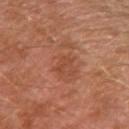notes: imaged on a skin check; not biopsied
subject: male, aged 28–32
location: the left upper arm
TBP lesion metrics: an area of roughly 2 mm² and an eccentricity of roughly 0.95; a border-irregularity rating of about 5.5/10, a within-lesion color-variation index near 0/10, and radial color variation of about 0
imaging modality: total-body-photography crop, ~15 mm field of view
lesion diameter: ~2.5 mm (longest diameter)
illumination: cross-polarized